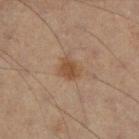Clinical impression:
Captured during whole-body skin photography for melanoma surveillance; the lesion was not biopsied.
Context:
The lesion is on the left leg. A roughly 15 mm field-of-view crop from a total-body skin photograph. The patient is a male approximately 50 years of age.